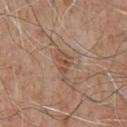workup: no biopsy performed (imaged during a skin exam) | subject: male, aged around 55 | image: 15 mm crop, total-body photography | lesion diameter: ≈3.5 mm | image-analysis metrics: a footprint of about 4 mm² and a shape eccentricity near 0.9; a mean CIELAB color near L≈50 a*≈18 b*≈28 and a lesion-to-skin contrast of about 6 (normalized; higher = more distinct); a color-variation rating of about 2.5/10 and peripheral color asymmetry of about 1 | body site: the chest.